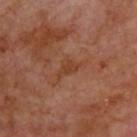diameter: ≈3 mm
illumination: cross-polarized
body site: the upper back
image-analysis metrics: a detector confidence of about 100 out of 100 that the crop contains a lesion
image source: total-body-photography crop, ~15 mm field of view
subject: male, aged 68–72